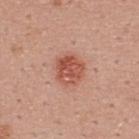Findings:
– notes: total-body-photography surveillance lesion; no biopsy
– patient: male, roughly 25 years of age
– location: the upper back
– image source: ~15 mm tile from a whole-body skin photo
– size: ≈3.5 mm